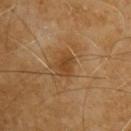The lesion was photographed on a routine skin check and not biopsied; there is no pathology result. A region of skin cropped from a whole-body photographic capture, roughly 15 mm wide. The lesion is on the upper back. A male patient aged 58–62. The tile uses cross-polarized illumination. An algorithmic analysis of the crop reported a lesion color around L≈40 a*≈19 b*≈35 in CIELAB, about 8 CIELAB-L* units darker than the surrounding skin, and a lesion-to-skin contrast of about 7 (normalized; higher = more distinct). And it measured a border-irregularity index near 3.5/10 and a within-lesion color-variation index near 2/10.